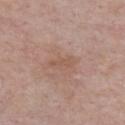| field | value |
|---|---|
| biopsy status | total-body-photography surveillance lesion; no biopsy |
| size | about 2.5 mm |
| location | the chest |
| TBP lesion metrics | a footprint of about 2.5 mm², an eccentricity of roughly 0.95, and two-axis asymmetry of about 0.4 |
| patient | male, aged 53–57 |
| tile lighting | white-light illumination |
| imaging modality | 15 mm crop, total-body photography |